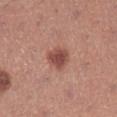Q: Is there a histopathology result?
A: imaged on a skin check; not biopsied
Q: Patient demographics?
A: female, aged around 30
Q: What is the anatomic site?
A: the right lower leg
Q: What is the imaging modality?
A: 15 mm crop, total-body photography